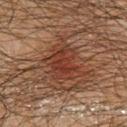Captured during whole-body skin photography for melanoma surveillance; the lesion was not biopsied. The lesion's longest dimension is about 4 mm. Captured under cross-polarized illumination. A roughly 15 mm field-of-view crop from a total-body skin photograph. The subject is a male about 60 years old. Located on the chest.